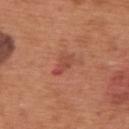Case summary:
- follow-up · total-body-photography surveillance lesion; no biopsy
- imaging modality · 15 mm crop, total-body photography
- automated lesion analysis · an area of roughly 3.5 mm², an outline eccentricity of about 0.9 (0 = round, 1 = elongated), and two-axis asymmetry of about 0.4; a mean CIELAB color near L≈48 a*≈29 b*≈31, roughly 8 lightness units darker than nearby skin, and a lesion-to-skin contrast of about 6 (normalized; higher = more distinct); a nevus-likeness score of about 0/100 and a detector confidence of about 100 out of 100 that the crop contains a lesion
- patient · male, roughly 65 years of age
- lighting · white-light
- location · the back
- lesion diameter · ≈3 mm